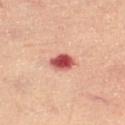Captured during whole-body skin photography for melanoma surveillance; the lesion was not biopsied.
A female subject in their mid- to late 40s.
The lesion is located on the leg.
This is a cross-polarized tile.
About 3 mm across.
A close-up tile cropped from a whole-body skin photograph, about 15 mm across.